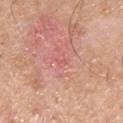follow-up: no biopsy performed (imaged during a skin exam) | diameter: ≈1.5 mm | patient: male, aged 53–57 | TBP lesion metrics: two-axis asymmetry of about 0.3; a lesion-to-skin contrast of about 3.5 (normalized; higher = more distinct); an automated nevus-likeness rating near 0 out of 100 and a detector confidence of about 85 out of 100 that the crop contains a lesion | lighting: white-light illumination | location: the front of the torso | acquisition: ~15 mm crop, total-body skin-cancer survey.